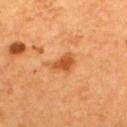workup: total-body-photography surveillance lesion; no biopsy | image: total-body-photography crop, ~15 mm field of view | patient: male, aged approximately 55 | location: the upper back | lighting: cross-polarized.